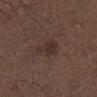workup: catalogued during a skin exam; not biopsied
acquisition: 15 mm crop, total-body photography
patient: male, about 50 years old
site: the left lower leg